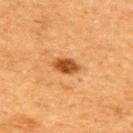Findings:
• follow-up — no biopsy performed (imaged during a skin exam)
• size — about 3 mm
• anatomic site — the right upper arm
• automated lesion analysis — a border-irregularity index near 1.5/10, a within-lesion color-variation index near 3.5/10, and peripheral color asymmetry of about 1.5; a nevus-likeness score of about 100/100
• image — ~15 mm crop, total-body skin-cancer survey
• patient — female, aged approximately 60
• lighting — cross-polarized